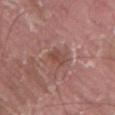Findings:
- size: about 2.5 mm
- illumination: white-light illumination
- site: the mid back
- subject: male, aged approximately 40
- image: ~15 mm crop, total-body skin-cancer survey